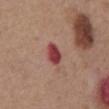The lesion was photographed on a routine skin check and not biopsied; there is no pathology result.
This is a white-light tile.
A close-up tile cropped from a whole-body skin photograph, about 15 mm across.
About 3 mm across.
The subject is a male about 75 years old.
Automated image analysis of the tile measured a nevus-likeness score of about 0/100 and lesion-presence confidence of about 100/100.
The lesion is on the front of the torso.